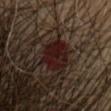Assessment:
Imaged during a routine full-body skin examination; the lesion was not biopsied and no histopathology is available.
Image and clinical context:
The total-body-photography lesion software estimated radial color variation of about 2.5. The software also gave a lesion-detection confidence of about 100/100. The patient is a female aged approximately 40. From the head or neck. Cropped from a whole-body photographic skin survey; the tile spans about 15 mm.